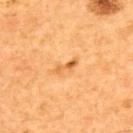Assessment:
Captured during whole-body skin photography for melanoma surveillance; the lesion was not biopsied.
Background:
A 15 mm close-up extracted from a 3D total-body photography capture. The subject is a male aged approximately 65. Measured at roughly 3 mm in maximum diameter. The lesion is on the upper back.